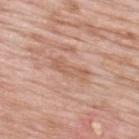follow-up: imaged on a skin check; not biopsied
subject: male, approximately 60 years of age
automated metrics: a lesion area of about 6 mm² and a shape eccentricity near 0.95; an average lesion color of about L≈60 a*≈21 b*≈30 (CIELAB), about 7 CIELAB-L* units darker than the surrounding skin, and a lesion-to-skin contrast of about 5.5 (normalized; higher = more distinct); a classifier nevus-likeness of about 0/100 and a lesion-detection confidence of about 90/100
illumination: white-light illumination
imaging modality: ~15 mm tile from a whole-body skin photo
size: about 4.5 mm
anatomic site: the back